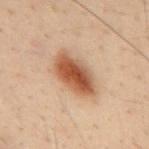Notes:
– notes — imaged on a skin check; not biopsied
– illumination — cross-polarized
– body site — the back
– image — ~15 mm crop, total-body skin-cancer survey
– TBP lesion metrics — border irregularity of about 2 on a 0–10 scale, a color-variation rating of about 6/10, and peripheral color asymmetry of about 1.5; a nevus-likeness score of about 100/100 and a lesion-detection confidence of about 100/100
– subject — male, aged 28–32
– diameter — ~5.5 mm (longest diameter)